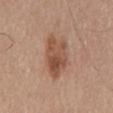Q: What are the patient's age and sex?
A: male, aged 63–67
Q: Illumination type?
A: white-light
Q: What kind of image is this?
A: total-body-photography crop, ~15 mm field of view
Q: Lesion location?
A: the back